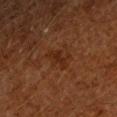| feature | finding |
|---|---|
| workup | no biopsy performed (imaged during a skin exam) |
| size | ~3.5 mm (longest diameter) |
| patient | male, aged 58 to 62 |
| tile lighting | cross-polarized |
| imaging modality | ~15 mm crop, total-body skin-cancer survey |
| body site | the left forearm |